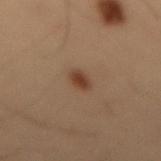notes: imaged on a skin check; not biopsied | patient: male, aged approximately 55 | diameter: about 2.5 mm | illumination: cross-polarized | image source: total-body-photography crop, ~15 mm field of view | body site: the lower back.